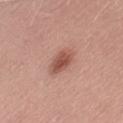  biopsy_status: not biopsied; imaged during a skin examination
  image:
    source: total-body photography crop
    field_of_view_mm: 15
  site: right thigh
  patient:
    sex: female
    age_approx: 55